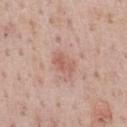The lesion was photographed on a routine skin check and not biopsied; there is no pathology result. The lesion is on the chest. About 2.5 mm across. Imaged with white-light lighting. A male subject, aged 73 to 77. An algorithmic analysis of the crop reported about 8 CIELAB-L* units darker than the surrounding skin and a lesion-to-skin contrast of about 5.5 (normalized; higher = more distinct). It also reported border irregularity of about 3 on a 0–10 scale, a color-variation rating of about 1/10, and a peripheral color-asymmetry measure near 0.5. A close-up tile cropped from a whole-body skin photograph, about 15 mm across.